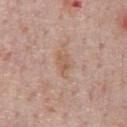follow-up=no biopsy performed (imaged during a skin exam).